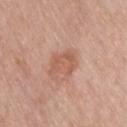• biopsy status: catalogued during a skin exam; not biopsied
• automated lesion analysis: a mean CIELAB color near L≈57 a*≈24 b*≈30, about 9 CIELAB-L* units darker than the surrounding skin, and a lesion-to-skin contrast of about 6 (normalized; higher = more distinct); internal color variation of about 2.5 on a 0–10 scale and peripheral color asymmetry of about 0.5; an automated nevus-likeness rating near 10 out of 100
• size: ≈4 mm
• body site: the upper back
• patient: male, about 60 years old
• lighting: white-light
• image source: total-body-photography crop, ~15 mm field of view